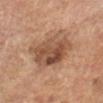<record>
<biopsy_status>not biopsied; imaged during a skin examination</biopsy_status>
<lesion_size>
  <long_diameter_mm_approx>6.0</long_diameter_mm_approx>
</lesion_size>
<patient>
  <sex>male</sex>
  <age_approx>55</age_approx>
</patient>
<image>
  <source>total-body photography crop</source>
  <field_of_view_mm>15</field_of_view_mm>
</image>
<site>left forearm</site>
</record>